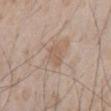Q: Was a biopsy performed?
A: catalogued during a skin exam; not biopsied
Q: What kind of image is this?
A: ~15 mm tile from a whole-body skin photo
Q: Lesion location?
A: the abdomen
Q: What did automated image analysis measure?
A: an area of roughly 4 mm², an outline eccentricity of about 0.75 (0 = round, 1 = elongated), and two-axis asymmetry of about 0.25; an average lesion color of about L≈58 a*≈16 b*≈28 (CIELAB), a lesion–skin lightness drop of about 6, and a normalized lesion–skin contrast near 5; a nevus-likeness score of about 0/100 and a lesion-detection confidence of about 100/100
Q: Who is the patient?
A: male, approximately 65 years of age